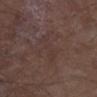Findings:
• follow-up — imaged on a skin check; not biopsied
• patient — male, in their mid- to late 70s
• body site — the left lower leg
• image source — ~15 mm crop, total-body skin-cancer survey
• size — ~4.5 mm (longest diameter)
• TBP lesion metrics — a shape eccentricity near 0.9